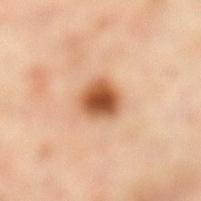workup — no biopsy performed (imaged during a skin exam) | diameter — about 3.5 mm | subject — female, roughly 55 years of age | location — the left lower leg | automated lesion analysis — an automated nevus-likeness rating near 100 out of 100 and lesion-presence confidence of about 100/100 | image source — total-body-photography crop, ~15 mm field of view | lighting — cross-polarized.